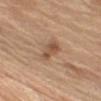notes: catalogued during a skin exam; not biopsied | lighting: white-light illumination | image: 15 mm crop, total-body photography | body site: the right upper arm | subject: male, aged approximately 70 | lesion size: ≈3.5 mm.